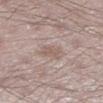{
  "biopsy_status": "not biopsied; imaged during a skin examination",
  "site": "leg",
  "image": {
    "source": "total-body photography crop",
    "field_of_view_mm": 15
  },
  "lesion_size": {
    "long_diameter_mm_approx": 2.5
  },
  "lighting": "white-light",
  "automated_metrics": {
    "cielab_L": 57,
    "cielab_a": 15,
    "cielab_b": 22,
    "vs_skin_contrast_norm": 5.5,
    "nevus_likeness_0_100": 20
  },
  "patient": {
    "sex": "male",
    "age_approx": 35
  }
}